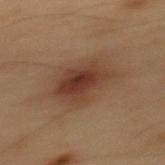Clinical impression:
Recorded during total-body skin imaging; not selected for excision or biopsy.
Background:
Imaged with cross-polarized lighting. A male patient, in their mid-50s. Measured at roughly 5 mm in maximum diameter. An algorithmic analysis of the crop reported an area of roughly 17 mm². It also reported a lesion color around L≈30 a*≈16 b*≈23 in CIELAB, roughly 8 lightness units darker than nearby skin, and a normalized lesion–skin contrast near 8.5. The software also gave a classifier nevus-likeness of about 95/100 and a detector confidence of about 100 out of 100 that the crop contains a lesion. The lesion is located on the mid back. A 15 mm crop from a total-body photograph taken for skin-cancer surveillance.